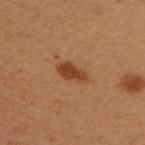Located on the left upper arm.
A female subject aged 48–52.
A 15 mm close-up tile from a total-body photography series done for melanoma screening.
This is a cross-polarized tile.